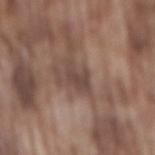Part of a total-body skin-imaging series; this lesion was reviewed on a skin check and was not flagged for biopsy. A close-up tile cropped from a whole-body skin photograph, about 15 mm across. About 3 mm across. The subject is a male aged approximately 75. From the mid back. Imaged with white-light lighting. The total-body-photography lesion software estimated border irregularity of about 4 on a 0–10 scale and internal color variation of about 2 on a 0–10 scale.